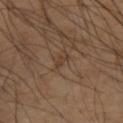Background: From the right upper arm. A male subject, approximately 50 years of age. Imaged with cross-polarized lighting. A 15 mm close-up tile from a total-body photography series done for melanoma screening.